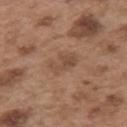Q: Was this lesion biopsied?
A: imaged on a skin check; not biopsied
Q: What kind of image is this?
A: 15 mm crop, total-body photography
Q: Lesion location?
A: the left upper arm
Q: Who is the patient?
A: male, aged 53 to 57